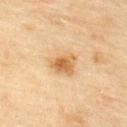Q: Is there a histopathology result?
A: catalogued during a skin exam; not biopsied
Q: How was the tile lit?
A: cross-polarized illumination
Q: What are the patient's age and sex?
A: male, aged approximately 45
Q: What is the imaging modality?
A: ~15 mm tile from a whole-body skin photo
Q: Lesion location?
A: the upper back
Q: What is the lesion's diameter?
A: about 4 mm